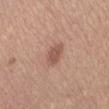follow-up = imaged on a skin check; not biopsied
acquisition = ~15 mm crop, total-body skin-cancer survey
automated lesion analysis = a classifier nevus-likeness of about 70/100 and a detector confidence of about 100 out of 100 that the crop contains a lesion
illumination = white-light illumination
site = the right thigh
size = about 2.5 mm
patient = female, aged 63 to 67